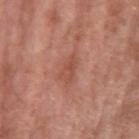follow-up = total-body-photography surveillance lesion; no biopsy
imaging modality = 15 mm crop, total-body photography
patient = male, aged around 80
site = the right forearm